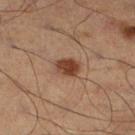notes = no biopsy performed (imaged during a skin exam)
acquisition = ~15 mm tile from a whole-body skin photo
subject = male, about 50 years old
tile lighting = cross-polarized illumination
lesion diameter = ~3 mm (longest diameter)
location = the left leg